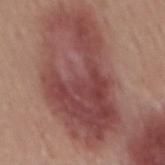Case summary:
* workup: no biopsy performed (imaged during a skin exam)
* illumination: white-light
* size: ≈12.5 mm
* body site: the mid back
* automated lesion analysis: a within-lesion color-variation index near 4.5/10; an automated nevus-likeness rating near 20 out of 100 and a detector confidence of about 100 out of 100 that the crop contains a lesion
* patient: male, in their mid-50s
* acquisition: ~15 mm crop, total-body skin-cancer survey